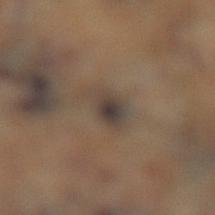<record>
  <biopsy_status>not biopsied; imaged during a skin examination</biopsy_status>
  <automated_metrics>
    <vs_skin_darker_L>8.0</vs_skin_darker_L>
    <vs_skin_contrast_norm>8.0</vs_skin_contrast_norm>
  </automated_metrics>
  <site>left lower leg</site>
  <lesion_size>
    <long_diameter_mm_approx>4.0</long_diameter_mm_approx>
  </lesion_size>
  <image>
    <source>total-body photography crop</source>
    <field_of_view_mm>15</field_of_view_mm>
  </image>
  <lighting>cross-polarized</lighting>
</record>